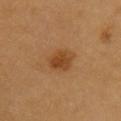Imaged during a routine full-body skin examination; the lesion was not biopsied and no histopathology is available. The lesion-visualizer software estimated an area of roughly 6.5 mm², a shape eccentricity near 0.55, and a symmetry-axis asymmetry near 0.2. And it measured roughly 9 lightness units darker than nearby skin and a lesion-to-skin contrast of about 7.5 (normalized; higher = more distinct). The software also gave an automated nevus-likeness rating near 95 out of 100 and a detector confidence of about 100 out of 100 that the crop contains a lesion. A male patient, aged around 60. Imaged with cross-polarized lighting. Located on the chest. Longest diameter approximately 3 mm. This image is a 15 mm lesion crop taken from a total-body photograph.